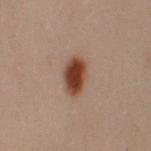{"site": "left arm", "image": {"source": "total-body photography crop", "field_of_view_mm": 15}, "patient": {"sex": "female", "age_approx": 30}}